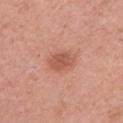Imaged during a routine full-body skin examination; the lesion was not biopsied and no histopathology is available. Located on the right upper arm. Cropped from a total-body skin-imaging series; the visible field is about 15 mm. Measured at roughly 3.5 mm in maximum diameter. The subject is a female approximately 35 years of age. The tile uses white-light illumination.